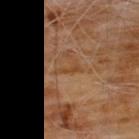Context:
Cropped from a total-body skin-imaging series; the visible field is about 15 mm. The subject is a male aged around 60. The lesion is located on the chest.